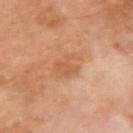- biopsy status: total-body-photography surveillance lesion; no biopsy
- lesion diameter: ~3.5 mm (longest diameter)
- image-analysis metrics: a lesion color around L≈56 a*≈23 b*≈36 in CIELAB, about 7 CIELAB-L* units darker than the surrounding skin, and a lesion-to-skin contrast of about 5 (normalized; higher = more distinct); a color-variation rating of about 2/10 and peripheral color asymmetry of about 1; a lesion-detection confidence of about 100/100
- site: the right upper arm
- acquisition: 15 mm crop, total-body photography
- patient: male, aged 68 to 72